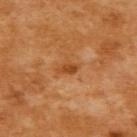Imaged during a routine full-body skin examination; the lesion was not biopsied and no histopathology is available. A region of skin cropped from a whole-body photographic capture, roughly 15 mm wide. This is a cross-polarized tile. A female subject, about 55 years old. Automated tile analysis of the lesion measured internal color variation of about 0.5 on a 0–10 scale and a peripheral color-asymmetry measure near 0.5. The analysis additionally found a detector confidence of about 100 out of 100 that the crop contains a lesion. Located on the upper back. Longest diameter approximately 2.5 mm.